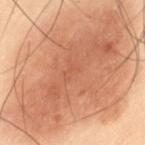<tbp_lesion>
<biopsy_status>not biopsied; imaged during a skin examination</biopsy_status>
</tbp_lesion>